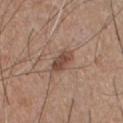follow-up: total-body-photography surveillance lesion; no biopsy | patient: male, aged approximately 55 | diameter: ≈3 mm | lighting: white-light illumination | body site: the chest | image source: 15 mm crop, total-body photography | image-analysis metrics: a footprint of about 5 mm² and a shape eccentricity near 0.8; a lesion color around L≈46 a*≈19 b*≈26 in CIELAB, roughly 11 lightness units darker than nearby skin, and a lesion-to-skin contrast of about 8 (normalized; higher = more distinct); a nevus-likeness score of about 55/100 and lesion-presence confidence of about 100/100.